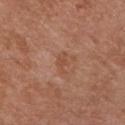Notes:
– follow-up — imaged on a skin check; not biopsied
– body site — the front of the torso
– lesion size — about 2.5 mm
– lighting — white-light illumination
– automated lesion analysis — a footprint of about 2.5 mm², a shape eccentricity near 0.85, and a shape-asymmetry score of about 0.35 (0 = symmetric); a lesion color around L≈49 a*≈23 b*≈32 in CIELAB, a lesion–skin lightness drop of about 7, and a lesion-to-skin contrast of about 5.5 (normalized; higher = more distinct); an automated nevus-likeness rating near 0 out of 100
– subject — male, approximately 55 years of age
– image source — total-body-photography crop, ~15 mm field of view